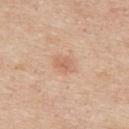The lesion was photographed on a routine skin check and not biopsied; there is no pathology result. The lesion-visualizer software estimated a mean CIELAB color near L≈64 a*≈22 b*≈32 and roughly 8 lightness units darker than nearby skin. And it measured a border-irregularity rating of about 2/10, a within-lesion color-variation index near 2/10, and a peripheral color-asymmetry measure near 0.5. The software also gave an automated nevus-likeness rating near 10 out of 100 and a detector confidence of about 100 out of 100 that the crop contains a lesion. The subject is a male aged approximately 55. Captured under white-light illumination. Longest diameter approximately 2.5 mm. This image is a 15 mm lesion crop taken from a total-body photograph. The lesion is located on the upper back.